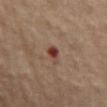Imaged during a routine full-body skin examination; the lesion was not biopsied and no histopathology is available.
The patient is a female approximately 65 years of age.
The lesion is on the abdomen.
A close-up tile cropped from a whole-body skin photograph, about 15 mm across.